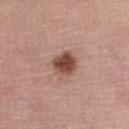notes: catalogued during a skin exam; not biopsied | subject: female, aged 63–67 | anatomic site: the left lower leg | image: ~15 mm tile from a whole-body skin photo | illumination: white-light illumination.